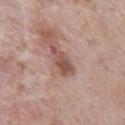Recorded during total-body skin imaging; not selected for excision or biopsy. The recorded lesion diameter is about 4 mm. The tile uses white-light illumination. Located on the chest. A 15 mm crop from a total-body photograph taken for skin-cancer surveillance. A male subject, aged around 70.